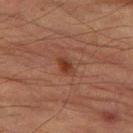Imaged during a routine full-body skin examination; the lesion was not biopsied and no histopathology is available.
Cropped from a total-body skin-imaging series; the visible field is about 15 mm.
Automated tile analysis of the lesion measured a footprint of about 3.5 mm², an eccentricity of roughly 0.8, and two-axis asymmetry of about 0.25. And it measured roughly 8 lightness units darker than nearby skin and a normalized border contrast of about 7.5. The analysis additionally found a border-irregularity rating of about 2.5/10, a within-lesion color-variation index near 2.5/10, and peripheral color asymmetry of about 0.5. The software also gave a lesion-detection confidence of about 100/100.
About 2.5 mm across.
A male subject, about 85 years old or older.
The tile uses cross-polarized illumination.
From the left thigh.